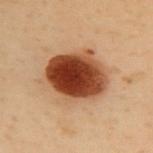The lesion was photographed on a routine skin check and not biopsied; there is no pathology result. A female patient roughly 60 years of age. A 15 mm crop from a total-body photograph taken for skin-cancer surveillance. From the upper back.